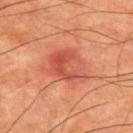Recorded during total-body skin imaging; not selected for excision or biopsy. A 15 mm close-up extracted from a 3D total-body photography capture. The subject is a male aged approximately 65. The lesion is located on the upper back.